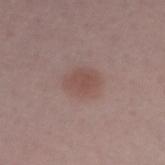Impression: Part of a total-body skin-imaging series; this lesion was reviewed on a skin check and was not flagged for biopsy. Background: The subject is a female about 45 years old. The tile uses white-light illumination. A roughly 15 mm field-of-view crop from a total-body skin photograph. Measured at roughly 3.5 mm in maximum diameter. The lesion is on the arm.